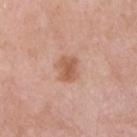{
  "biopsy_status": "not biopsied; imaged during a skin examination",
  "site": "chest",
  "patient": {
    "sex": "male",
    "age_approx": 55
  },
  "image": {
    "source": "total-body photography crop",
    "field_of_view_mm": 15
  }
}